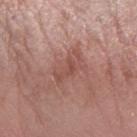Clinical impression:
No biopsy was performed on this lesion — it was imaged during a full skin examination and was not determined to be concerning.
Context:
The lesion is located on the left forearm. Cropped from a whole-body photographic skin survey; the tile spans about 15 mm. A female subject aged approximately 70. The recorded lesion diameter is about 4 mm. The total-body-photography lesion software estimated a footprint of about 6.5 mm² and a symmetry-axis asymmetry near 0.4. And it measured a nevus-likeness score of about 0/100 and lesion-presence confidence of about 100/100.